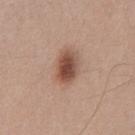No biopsy was performed on this lesion — it was imaged during a full skin examination and was not determined to be concerning.
Captured under white-light illumination.
Measured at roughly 4 mm in maximum diameter.
This image is a 15 mm lesion crop taken from a total-body photograph.
The patient is a male in their mid- to late 20s.
On the abdomen.